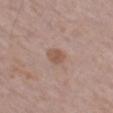<tbp_lesion>
  <biopsy_status>not biopsied; imaged during a skin examination</biopsy_status>
  <site>leg</site>
  <lesion_size>
    <long_diameter_mm_approx>2.5</long_diameter_mm_approx>
  </lesion_size>
  <patient>
    <sex>male</sex>
    <age_approx>80</age_approx>
  </patient>
  <image>
    <source>total-body photography crop</source>
    <field_of_view_mm>15</field_of_view_mm>
  </image>
  <lighting>white-light</lighting>
</tbp_lesion>